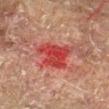Clinical impression: The lesion was tiled from a total-body skin photograph and was not biopsied. Acquisition and patient details: A 15 mm close-up extracted from a 3D total-body photography capture. The lesion is on the arm. The patient is a male about 60 years old. Measured at roughly 4.5 mm in maximum diameter.